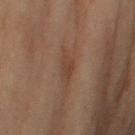| field | value |
|---|---|
| workup | catalogued during a skin exam; not biopsied |
| location | the right upper arm |
| patient | male, roughly 70 years of age |
| lesion size | about 2.5 mm |
| acquisition | total-body-photography crop, ~15 mm field of view |